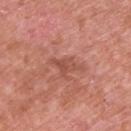The subject is a male about 70 years old. The lesion's longest dimension is about 3 mm. A close-up tile cropped from a whole-body skin photograph, about 15 mm across. The lesion is on the upper back. Automated tile analysis of the lesion measured an area of roughly 4 mm², an outline eccentricity of about 0.75 (0 = round, 1 = elongated), and two-axis asymmetry of about 0.45. The software also gave an average lesion color of about L≈51 a*≈27 b*≈29 (CIELAB) and a normalized lesion–skin contrast near 5.5. The analysis additionally found a border-irregularity rating of about 4.5/10, internal color variation of about 1.5 on a 0–10 scale, and a peripheral color-asymmetry measure near 0.5. Imaged with white-light lighting.